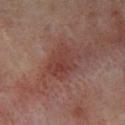biopsy_status: not biopsied; imaged during a skin examination
automated_metrics:
  area_mm2_approx: 11.0
  eccentricity: 0.75
  shape_asymmetry: 0.2
  border_irregularity_0_10: 3.0
  color_variation_0_10: 4.0
  peripheral_color_asymmetry: 1.5
patient:
  sex: female
  age_approx: 50
image:
  source: total-body photography crop
  field_of_view_mm: 15
site: right lower leg
lighting: cross-polarized